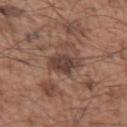biopsy status — imaged on a skin check; not biopsied | acquisition — 15 mm crop, total-body photography | subject — male, aged 53 to 57 | anatomic site — the arm.